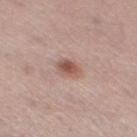biopsy_status: not biopsied; imaged during a skin examination
patient:
  sex: female
  age_approx: 45
image:
  source: total-body photography crop
  field_of_view_mm: 15
lesion_size:
  long_diameter_mm_approx: 3.0
site: right thigh
lighting: white-light